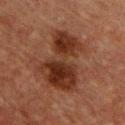Clinical impression:
Recorded during total-body skin imaging; not selected for excision or biopsy.